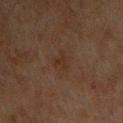The lesion was photographed on a routine skin check and not biopsied; there is no pathology result.
From the upper back.
The tile uses cross-polarized illumination.
An algorithmic analysis of the crop reported a footprint of about 4 mm², a shape eccentricity near 0.6, and a symmetry-axis asymmetry near 0.45. It also reported a lesion-detection confidence of about 100/100.
A male subject, about 65 years old.
This image is a 15 mm lesion crop taken from a total-body photograph.